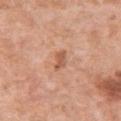{"biopsy_status": "not biopsied; imaged during a skin examination", "site": "chest", "lighting": "white-light", "lesion_size": {"long_diameter_mm_approx": 2.5}, "patient": {"sex": "female", "age_approx": 50}, "image": {"source": "total-body photography crop", "field_of_view_mm": 15}}